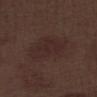Impression: Imaged during a routine full-body skin examination; the lesion was not biopsied and no histopathology is available. Clinical summary: From the left lower leg. Cropped from a whole-body photographic skin survey; the tile spans about 15 mm. The subject is a male aged 68 to 72. The total-body-photography lesion software estimated a lesion area of about 20 mm², a shape eccentricity near 0.7, and a symmetry-axis asymmetry near 0.2. The software also gave a mean CIELAB color near L≈26 a*≈15 b*≈17 and a normalized lesion–skin contrast near 5. The analysis additionally found a border-irregularity rating of about 2.5/10 and a color-variation rating of about 2/10. This is a white-light tile.